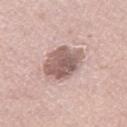- notes · imaged on a skin check; not biopsied
- lighting · white-light illumination
- automated lesion analysis · a shape eccentricity near 0.6 and a symmetry-axis asymmetry near 0.15; a lesion color around L≈58 a*≈18 b*≈22 in CIELAB, a lesion–skin lightness drop of about 14, and a normalized lesion–skin contrast near 9
- location · the leg
- imaging modality · 15 mm crop, total-body photography
- diameter · ≈5 mm
- patient · male, roughly 65 years of age